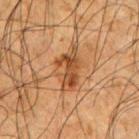workup = total-body-photography surveillance lesion; no biopsy | image = total-body-photography crop, ~15 mm field of view | subject = male, aged around 65 | anatomic site = the arm.